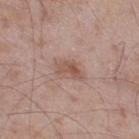notes: no biopsy performed (imaged during a skin exam) | acquisition: 15 mm crop, total-body photography | anatomic site: the right thigh | patient: male, in their mid- to late 50s | illumination: white-light | lesion diameter: ≈3 mm | TBP lesion metrics: an area of roughly 4.5 mm², an outline eccentricity of about 0.8 (0 = round, 1 = elongated), and two-axis asymmetry of about 0.45; an average lesion color of about L≈53 a*≈20 b*≈26 (CIELAB), roughly 9 lightness units darker than nearby skin, and a lesion-to-skin contrast of about 6.5 (normalized; higher = more distinct); a border-irregularity index near 5/10 and a within-lesion color-variation index near 4/10.